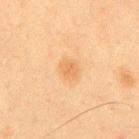Captured during whole-body skin photography for melanoma surveillance; the lesion was not biopsied.
A male subject aged approximately 35.
A roughly 15 mm field-of-view crop from a total-body skin photograph.
On the back.